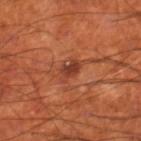Clinical impression: Captured during whole-body skin photography for melanoma surveillance; the lesion was not biopsied. Acquisition and patient details: The lesion's longest dimension is about 3 mm. The lesion is located on the right thigh. Imaged with cross-polarized lighting. The patient is a male aged 68 to 72. Cropped from a total-body skin-imaging series; the visible field is about 15 mm.